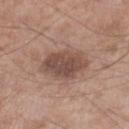notes: catalogued during a skin exam; not biopsied | lesion size: about 5.5 mm | subject: male, roughly 55 years of age | lighting: white-light illumination | image source: 15 mm crop, total-body photography | location: the right lower leg.